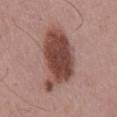Assessment:
This lesion was catalogued during total-body skin photography and was not selected for biopsy.
Background:
Cropped from a total-body skin-imaging series; the visible field is about 15 mm. An algorithmic analysis of the crop reported a footprint of about 25 mm² and two-axis asymmetry of about 0.3. The analysis additionally found an average lesion color of about L≈45 a*≈22 b*≈23 (CIELAB) and roughly 16 lightness units darker than nearby skin. And it measured a color-variation rating of about 4.5/10 and peripheral color asymmetry of about 1.5. Imaged with white-light lighting. The lesion is on the mid back. The lesion's longest dimension is about 7.5 mm. A male subject aged 53 to 57.